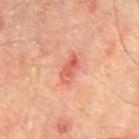<case>
<biopsy_status>not biopsied; imaged during a skin examination</biopsy_status>
<lighting>cross-polarized</lighting>
<patient>
  <sex>male</sex>
  <age_approx>65</age_approx>
</patient>
<image>
  <source>total-body photography crop</source>
  <field_of_view_mm>15</field_of_view_mm>
</image>
<site>back</site>
</case>